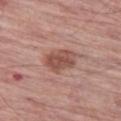workup: total-body-photography surveillance lesion; no biopsy
automated lesion analysis: an average lesion color of about L≈51 a*≈22 b*≈26 (CIELAB) and a normalized lesion–skin contrast near 7.5; a border-irregularity index near 2/10
lesion diameter: ≈4.5 mm
subject: male, aged approximately 75
tile lighting: white-light illumination
acquisition: total-body-photography crop, ~15 mm field of view
site: the left thigh